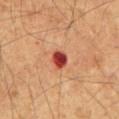A male subject aged around 60. Cropped from a whole-body photographic skin survey; the tile spans about 15 mm. The recorded lesion diameter is about 2.5 mm. The lesion is located on the back.